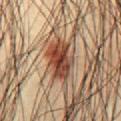<record>
  <biopsy_status>not biopsied; imaged during a skin examination</biopsy_status>
  <lesion_size>
    <long_diameter_mm_approx>5.0</long_diameter_mm_approx>
  </lesion_size>
  <image>
    <source>total-body photography crop</source>
    <field_of_view_mm>15</field_of_view_mm>
  </image>
  <site>abdomen</site>
  <patient>
    <sex>male</sex>
    <age_approx>35</age_approx>
  </patient>
</record>